A male patient, roughly 55 years of age; a close-up tile cropped from a whole-body skin photograph, about 15 mm across; from the arm: 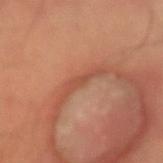Clinical summary:
Approximately 4.5 mm at its widest. The lesion-visualizer software estimated a lesion area of about 3.5 mm², an outline eccentricity of about 0.95 (0 = round, 1 = elongated), and a shape-asymmetry score of about 0.6 (0 = symmetric).
Diagnosis:
Histopathologically confirmed as a seborrheic keratosis.